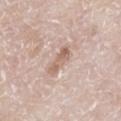notes — no biopsy performed (imaged during a skin exam)
subject — male, aged 78 to 82
lesion diameter — about 3.5 mm
location — the right lower leg
tile lighting — white-light illumination
image source — ~15 mm crop, total-body skin-cancer survey
TBP lesion metrics — a border-irregularity rating of about 3.5/10 and a within-lesion color-variation index near 3/10